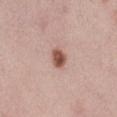Captured during whole-body skin photography for melanoma surveillance; the lesion was not biopsied.